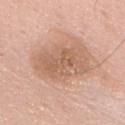Captured during whole-body skin photography for melanoma surveillance; the lesion was not biopsied. A male patient, aged around 60. The lesion is on the upper back. Longest diameter approximately 7 mm. Cropped from a total-body skin-imaging series; the visible field is about 15 mm.